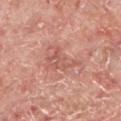A male patient, roughly 65 years of age. Located on the left lower leg. A close-up tile cropped from a whole-body skin photograph, about 15 mm across. Longest diameter approximately 5 mm. The tile uses white-light illumination.